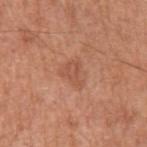Clinical impression:
No biopsy was performed on this lesion — it was imaged during a full skin examination and was not determined to be concerning.
Context:
The patient is a male aged approximately 65. The lesion is located on the left upper arm. A roughly 15 mm field-of-view crop from a total-body skin photograph.